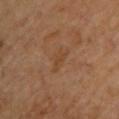The lesion was photographed on a routine skin check and not biopsied; there is no pathology result. This is a cross-polarized tile. A female subject, approximately 70 years of age. Cropped from a total-body skin-imaging series; the visible field is about 15 mm. An algorithmic analysis of the crop reported an area of roughly 3.5 mm², an outline eccentricity of about 0.8 (0 = round, 1 = elongated), and two-axis asymmetry of about 0.45. And it measured a border-irregularity index near 4.5/10, a color-variation rating of about 1/10, and a peripheral color-asymmetry measure near 0.5. Located on the upper back.